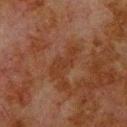Q: Was a biopsy performed?
A: imaged on a skin check; not biopsied
Q: How was this image acquired?
A: ~15 mm crop, total-body skin-cancer survey
Q: Patient demographics?
A: male, approximately 80 years of age
Q: Lesion size?
A: ≈4.5 mm
Q: Where on the body is the lesion?
A: the upper back